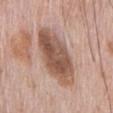Imaged during a routine full-body skin examination; the lesion was not biopsied and no histopathology is available. A male subject, in their 70s. Captured under white-light illumination. From the chest. A roughly 15 mm field-of-view crop from a total-body skin photograph. An algorithmic analysis of the crop reported an outline eccentricity of about 0.85 (0 = round, 1 = elongated). The analysis additionally found an automated nevus-likeness rating near 10 out of 100.